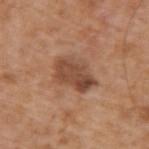notes: catalogued during a skin exam; not biopsied
acquisition: ~15 mm crop, total-body skin-cancer survey
image-analysis metrics: an area of roughly 12 mm², an eccentricity of roughly 0.8, and two-axis asymmetry of about 0.25; roughly 11 lightness units darker than nearby skin; a color-variation rating of about 4.5/10 and radial color variation of about 1.5
patient: male, approximately 65 years of age
body site: the upper back
illumination: white-light illumination
lesion size: ≈5 mm